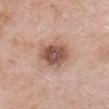<case>
<biopsy_status>not biopsied; imaged during a skin examination</biopsy_status>
<image>
  <source>total-body photography crop</source>
  <field_of_view_mm>15</field_of_view_mm>
</image>
<site>chest</site>
<patient>
  <sex>female</sex>
  <age_approx>70</age_approx>
</patient>
</case>